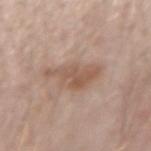Clinical impression: Captured during whole-body skin photography for melanoma surveillance; the lesion was not biopsied. Clinical summary: An algorithmic analysis of the crop reported an area of roughly 9.5 mm², a shape eccentricity near 0.8, and a shape-asymmetry score of about 0.45 (0 = symmetric). The analysis additionally found a lesion color around L≈55 a*≈18 b*≈27 in CIELAB, roughly 9 lightness units darker than nearby skin, and a normalized border contrast of about 6.5. The software also gave border irregularity of about 6 on a 0–10 scale, a color-variation rating of about 3/10, and a peripheral color-asymmetry measure near 1. Located on the right forearm. The tile uses white-light illumination. A male patient, approximately 40 years of age. The lesion's longest dimension is about 5 mm. This image is a 15 mm lesion crop taken from a total-body photograph.